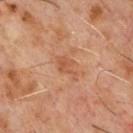This lesion was catalogued during total-body skin photography and was not selected for biopsy.